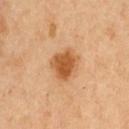notes: total-body-photography surveillance lesion; no biopsy
tile lighting: cross-polarized
size: ≈4 mm
body site: the front of the torso
imaging modality: ~15 mm crop, total-body skin-cancer survey
subject: male, roughly 50 years of age
image-analysis metrics: an area of roughly 10 mm² and a shape-asymmetry score of about 0.2 (0 = symmetric); a mean CIELAB color near L≈56 a*≈25 b*≈41, a lesion–skin lightness drop of about 13, and a normalized border contrast of about 9; a within-lesion color-variation index near 3.5/10 and peripheral color asymmetry of about 1; an automated nevus-likeness rating near 100 out of 100